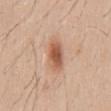Impression:
The lesion was photographed on a routine skin check and not biopsied; there is no pathology result.
Acquisition and patient details:
The lesion's longest dimension is about 4 mm. A lesion tile, about 15 mm wide, cut from a 3D total-body photograph. Captured under white-light illumination. The lesion is on the lower back. A male subject about 35 years old.